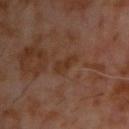Q: What is the imaging modality?
A: ~15 mm tile from a whole-body skin photo
Q: Lesion location?
A: the upper back
Q: What are the patient's age and sex?
A: male, roughly 60 years of age
Q: Lesion size?
A: about 3 mm